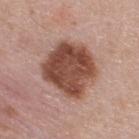Findings:
- workup — total-body-photography surveillance lesion; no biopsy
- image-analysis metrics — two-axis asymmetry of about 0.15; border irregularity of about 1.5 on a 0–10 scale, a within-lesion color-variation index near 4.5/10, and a peripheral color-asymmetry measure near 1.5; an automated nevus-likeness rating near 5 out of 100 and lesion-presence confidence of about 100/100
- illumination — white-light
- subject — male, aged around 45
- site — the upper back
- lesion size — ~6 mm (longest diameter)
- acquisition — total-body-photography crop, ~15 mm field of view